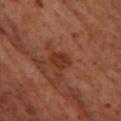{
  "biopsy_status": "not biopsied; imaged during a skin examination",
  "site": "left thigh",
  "patient": {
    "sex": "male",
    "age_approx": 60
  },
  "image": {
    "source": "total-body photography crop",
    "field_of_view_mm": 15
  }
}